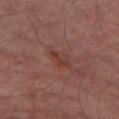Assessment: Part of a total-body skin-imaging series; this lesion was reviewed on a skin check and was not flagged for biopsy. Acquisition and patient details: A male subject aged approximately 65. A lesion tile, about 15 mm wide, cut from a 3D total-body photograph. The lesion is on the left thigh.